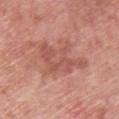Clinical impression: Imaged during a routine full-body skin examination; the lesion was not biopsied and no histopathology is available. Context: A male patient, aged 58–62. Cropped from a whole-body photographic skin survey; the tile spans about 15 mm. On the upper back. The total-body-photography lesion software estimated an average lesion color of about L≈54 a*≈26 b*≈27 (CIELAB), a lesion–skin lightness drop of about 9, and a normalized border contrast of about 6. The software also gave a classifier nevus-likeness of about 0/100. This is a white-light tile.